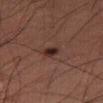biopsy status: imaged on a skin check; not biopsied
automated metrics: an area of roughly 3 mm², a shape eccentricity near 0.85, and a shape-asymmetry score of about 0.25 (0 = symmetric); a normalized lesion–skin contrast near 10.5; border irregularity of about 2.5 on a 0–10 scale and a within-lesion color-variation index near 3.5/10; a lesion-detection confidence of about 100/100
diameter: about 2.5 mm
subject: male, aged around 55
anatomic site: the left thigh
image: ~15 mm crop, total-body skin-cancer survey
illumination: cross-polarized illumination